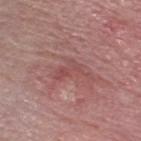Imaged during a routine full-body skin examination; the lesion was not biopsied and no histopathology is available. The lesion is located on the head or neck. A male patient, approximately 70 years of age. The tile uses white-light illumination. Cropped from a whole-body photographic skin survey; the tile spans about 15 mm. The recorded lesion diameter is about 4 mm. Automated image analysis of the tile measured a border-irregularity index near 7/10 and radial color variation of about 1.